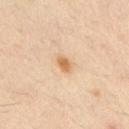Q: Was this lesion biopsied?
A: imaged on a skin check; not biopsied
Q: What kind of image is this?
A: ~15 mm tile from a whole-body skin photo
Q: How large is the lesion?
A: ~2 mm (longest diameter)
Q: What lighting was used for the tile?
A: cross-polarized
Q: Lesion location?
A: the right upper arm
Q: Patient demographics?
A: male, aged approximately 45